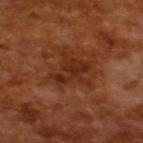This lesion was catalogued during total-body skin photography and was not selected for biopsy.
Imaged with cross-polarized lighting.
The patient is a male aged 63 to 67.
A 15 mm crop from a total-body photograph taken for skin-cancer surveillance.
Automated image analysis of the tile measured a symmetry-axis asymmetry near 0.45. The software also gave a mean CIELAB color near L≈29 a*≈24 b*≈31, a lesion–skin lightness drop of about 7, and a normalized border contrast of about 7. It also reported a detector confidence of about 100 out of 100 that the crop contains a lesion.